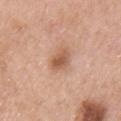The lesion was photographed on a routine skin check and not biopsied; there is no pathology result.
The lesion-visualizer software estimated a lesion area of about 5.5 mm², an eccentricity of roughly 0.8, and a shape-asymmetry score of about 0.2 (0 = symmetric). And it measured a normalized border contrast of about 7.5. It also reported internal color variation of about 4 on a 0–10 scale and a peripheral color-asymmetry measure near 1.5.
A 15 mm close-up tile from a total-body photography series done for melanoma screening.
Captured under white-light illumination.
A male patient, in their mid-50s.
Located on the chest.